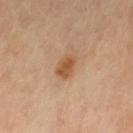Assessment:
The lesion was tiled from a total-body skin photograph and was not biopsied.
Context:
On the right leg. A female subject, about 60 years old. This is a cross-polarized tile. A region of skin cropped from a whole-body photographic capture, roughly 15 mm wide.